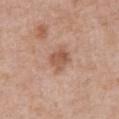Q: Is there a histopathology result?
A: total-body-photography surveillance lesion; no biopsy
Q: Patient demographics?
A: male, about 70 years old
Q: What is the imaging modality?
A: 15 mm crop, total-body photography
Q: Automated lesion metrics?
A: roughly 10 lightness units darker than nearby skin and a lesion-to-skin contrast of about 7 (normalized; higher = more distinct); radial color variation of about 1.5; a classifier nevus-likeness of about 5/100 and a lesion-detection confidence of about 100/100
Q: Illumination type?
A: white-light illumination
Q: Where on the body is the lesion?
A: the abdomen